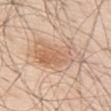{
  "biopsy_status": "not biopsied; imaged during a skin examination",
  "image": {
    "source": "total-body photography crop",
    "field_of_view_mm": 15
  },
  "site": "upper back",
  "patient": {
    "sex": "male",
    "age_approx": 80
  }
}